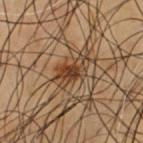Clinical summary:
The lesion is on the chest. The recorded lesion diameter is about 4 mm. A roughly 15 mm field-of-view crop from a total-body skin photograph. An algorithmic analysis of the crop reported a shape eccentricity near 0.8. And it measured an average lesion color of about L≈32 a*≈15 b*≈27 (CIELAB), a lesion–skin lightness drop of about 9, and a normalized border contrast of about 8.5. The software also gave an automated nevus-likeness rating near 100 out of 100. A male patient aged approximately 55. Captured under cross-polarized illumination.